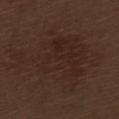workup: total-body-photography surveillance lesion; no biopsy | site: the left thigh | image source: ~15 mm tile from a whole-body skin photo | patient: male, roughly 70 years of age.